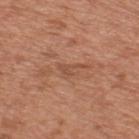The lesion was tiled from a total-body skin photograph and was not biopsied. Captured under white-light illumination. From the back. A male patient, aged approximately 65. The recorded lesion diameter is about 3 mm. A lesion tile, about 15 mm wide, cut from a 3D total-body photograph.